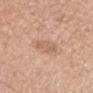Case summary:
• workup · imaged on a skin check; not biopsied
• image source · ~15 mm tile from a whole-body skin photo
• subject · female, aged 38 to 42
• tile lighting · white-light
• TBP lesion metrics · lesion-presence confidence of about 100/100
• site · the head or neck